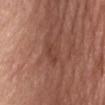No biopsy was performed on this lesion — it was imaged during a full skin examination and was not determined to be concerning. A 15 mm close-up extracted from a 3D total-body photography capture. This is a white-light tile. A male subject approximately 70 years of age. The lesion is on the left thigh. Approximately 3 mm at its widest. Automated image analysis of the tile measured about 6 CIELAB-L* units darker than the surrounding skin and a normalized lesion–skin contrast near 5. And it measured a border-irregularity index near 2/10, internal color variation of about 2.5 on a 0–10 scale, and radial color variation of about 1. It also reported a classifier nevus-likeness of about 0/100 and a detector confidence of about 100 out of 100 that the crop contains a lesion.